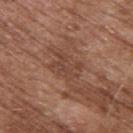Clinical impression:
Recorded during total-body skin imaging; not selected for excision or biopsy.
Context:
From the upper back. Approximately 4 mm at its widest. A roughly 15 mm field-of-view crop from a total-body skin photograph. Imaged with white-light lighting. A male patient aged 73 to 77.